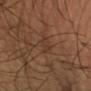workup=catalogued during a skin exam; not biopsied
body site=the left forearm
TBP lesion metrics=an area of roughly 3 mm²
acquisition=~15 mm tile from a whole-body skin photo
lesion diameter=about 2.5 mm
subject=male, about 45 years old
tile lighting=cross-polarized